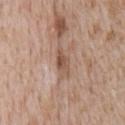notes = no biopsy performed (imaged during a skin exam) | automated lesion analysis = an area of roughly 5 mm², an eccentricity of roughly 0.9, and two-axis asymmetry of about 0.25; about 10 CIELAB-L* units darker than the surrounding skin; a border-irregularity rating of about 2.5/10, a color-variation rating of about 4/10, and a peripheral color-asymmetry measure near 1 | diameter = ≈3.5 mm | body site = the chest | image = ~15 mm crop, total-body skin-cancer survey | patient = male, aged around 65.